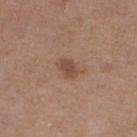Q: Is there a histopathology result?
A: catalogued during a skin exam; not biopsied
Q: Patient demographics?
A: female, approximately 45 years of age
Q: How was the tile lit?
A: white-light
Q: How was this image acquired?
A: 15 mm crop, total-body photography
Q: Automated lesion metrics?
A: a footprint of about 4 mm² and a symmetry-axis asymmetry near 0.25; a border-irregularity index near 2.5/10, a color-variation rating of about 2/10, and radial color variation of about 0.5; a nevus-likeness score of about 75/100
Q: Where on the body is the lesion?
A: the leg